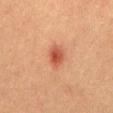The tile uses cross-polarized illumination.
Approximately 3 mm at its widest.
The subject is a male aged approximately 40.
The lesion is on the back.
A lesion tile, about 15 mm wide, cut from a 3D total-body photograph.